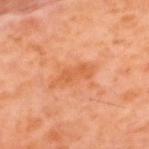| key | value |
|---|---|
| workup | imaged on a skin check; not biopsied |
| subject | male, about 60 years old |
| anatomic site | the upper back |
| diameter | ≈4.5 mm |
| image source | 15 mm crop, total-body photography |
| automated metrics | a footprint of about 6.5 mm² and a symmetry-axis asymmetry near 0.35; a lesion color around L≈56 a*≈28 b*≈40 in CIELAB, roughly 7 lightness units darker than nearby skin, and a normalized border contrast of about 6; border irregularity of about 4.5 on a 0–10 scale; a lesion-detection confidence of about 100/100 |
| tile lighting | cross-polarized illumination |